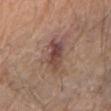biopsy status = total-body-photography surveillance lesion; no biopsy | imaging modality = ~15 mm tile from a whole-body skin photo | subject = male, aged approximately 70 | anatomic site = the arm.